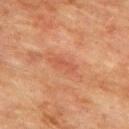No biopsy was performed on this lesion — it was imaged during a full skin examination and was not determined to be concerning. A 15 mm close-up extracted from a 3D total-body photography capture. The lesion is on the upper back. Automated tile analysis of the lesion measured a lesion area of about 3 mm², an eccentricity of roughly 0.9, and two-axis asymmetry of about 0.4. The analysis additionally found an average lesion color of about L≈45 a*≈26 b*≈30 (CIELAB), a lesion–skin lightness drop of about 6, and a lesion-to-skin contrast of about 4.5 (normalized; higher = more distinct). The lesion's longest dimension is about 2.5 mm. This is a cross-polarized tile. The subject is a male about 75 years old.